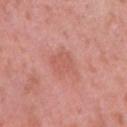biopsy status — imaged on a skin check; not biopsied
body site — the right upper arm
patient — female, approximately 40 years of age
acquisition — ~15 mm crop, total-body skin-cancer survey
illumination — white-light illumination
lesion size — ≈3.5 mm
image-analysis metrics — a footprint of about 8 mm² and an outline eccentricity of about 0.3 (0 = round, 1 = elongated); an average lesion color of about L≈58 a*≈28 b*≈28 (CIELAB) and a lesion-to-skin contrast of about 4.5 (normalized; higher = more distinct); a color-variation rating of about 1.5/10 and peripheral color asymmetry of about 0.5; a classifier nevus-likeness of about 0/100 and lesion-presence confidence of about 100/100